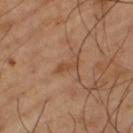| feature | finding |
|---|---|
| biopsy status | imaged on a skin check; not biopsied |
| subject | male, approximately 65 years of age |
| site | the left thigh |
| acquisition | ~15 mm crop, total-body skin-cancer survey |
| tile lighting | cross-polarized illumination |
| automated metrics | a shape eccentricity near 0.95 and a symmetry-axis asymmetry near 0.4; a lesion color around L≈46 a*≈22 b*≈33 in CIELAB and a normalized lesion–skin contrast near 6.5 |
| diameter | about 2.5 mm |